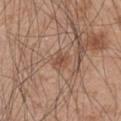Clinical impression:
Imaged during a routine full-body skin examination; the lesion was not biopsied and no histopathology is available.
Context:
This is a white-light tile. The lesion is on the arm. A male patient, aged 43 to 47. About 2.5 mm across. Cropped from a total-body skin-imaging series; the visible field is about 15 mm.